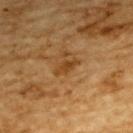From the back. The subject is a male in their mid- to late 80s or older. A close-up tile cropped from a whole-body skin photograph, about 15 mm across.